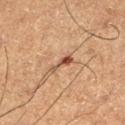- workup: total-body-photography surveillance lesion; no biopsy
- lighting: cross-polarized illumination
- patient: male, aged 53–57
- lesion diameter: about 3 mm
- image source: ~15 mm crop, total-body skin-cancer survey
- site: the left lower leg